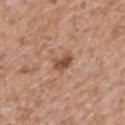Q: Automated lesion metrics?
A: an area of roughly 4 mm² and a symmetry-axis asymmetry near 0.3; an average lesion color of about L≈50 a*≈23 b*≈31 (CIELAB) and a normalized border contrast of about 8.5
Q: What lighting was used for the tile?
A: white-light illumination
Q: Patient demographics?
A: male, aged approximately 65
Q: What is the lesion's diameter?
A: about 2.5 mm
Q: How was this image acquired?
A: ~15 mm tile from a whole-body skin photo
Q: What is the anatomic site?
A: the back